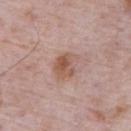Clinical summary: Automated tile analysis of the lesion measured an average lesion color of about L≈55 a*≈20 b*≈27 (CIELAB) and a normalized lesion–skin contrast near 7.5. The analysis additionally found border irregularity of about 3 on a 0–10 scale and internal color variation of about 5 on a 0–10 scale. A male subject aged around 70. Measured at roughly 3.5 mm in maximum diameter. On the abdomen. A roughly 15 mm field-of-view crop from a total-body skin photograph. This is a white-light tile.